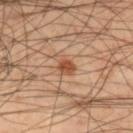Q: Was this lesion biopsied?
A: total-body-photography surveillance lesion; no biopsy
Q: What kind of image is this?
A: ~15 mm tile from a whole-body skin photo
Q: Where on the body is the lesion?
A: the leg
Q: Patient demographics?
A: male, aged approximately 45